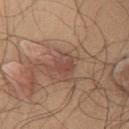The lesion was tiled from a total-body skin photograph and was not biopsied.
This image is a 15 mm lesion crop taken from a total-body photograph.
A male patient, aged around 45.
On the chest.
The lesion's longest dimension is about 3 mm.
The lesion-visualizer software estimated an eccentricity of roughly 0.6 and a symmetry-axis asymmetry near 0.3. The software also gave a detector confidence of about 100 out of 100 that the crop contains a lesion.